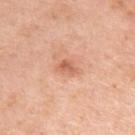notes = catalogued during a skin exam; not biopsied | automated lesion analysis = an automated nevus-likeness rating near 5 out of 100 and a lesion-detection confidence of about 100/100 | lighting = white-light | anatomic site = the left upper arm | image = 15 mm crop, total-body photography | size = about 2.5 mm | subject = female, about 40 years old.